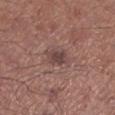This lesion was catalogued during total-body skin photography and was not selected for biopsy.
The lesion is located on the left lower leg.
A male patient aged 68–72.
A roughly 15 mm field-of-view crop from a total-body skin photograph.
The tile uses white-light illumination.
Automated tile analysis of the lesion measured a lesion color around L≈43 a*≈18 b*≈19 in CIELAB, roughly 9 lightness units darker than nearby skin, and a lesion-to-skin contrast of about 7.5 (normalized; higher = more distinct). It also reported a peripheral color-asymmetry measure near 1. The software also gave a classifier nevus-likeness of about 15/100.
About 2.5 mm across.